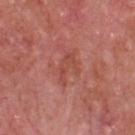| feature | finding |
|---|---|
| follow-up | catalogued during a skin exam; not biopsied |
| automated metrics | a lesion area of about 5.5 mm² and an eccentricity of roughly 0.85; a classifier nevus-likeness of about 0/100 |
| image | 15 mm crop, total-body photography |
| anatomic site | the chest |
| subject | male, aged around 70 |
| tile lighting | white-light illumination |